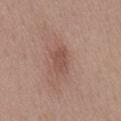Assessment:
Captured during whole-body skin photography for melanoma surveillance; the lesion was not biopsied.
Context:
Cropped from a whole-body photographic skin survey; the tile spans about 15 mm. The lesion is on the mid back. This is a white-light tile. A female subject aged 48–52. The recorded lesion diameter is about 4 mm.